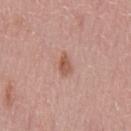Impression:
The lesion was photographed on a routine skin check and not biopsied; there is no pathology result.
Image and clinical context:
The recorded lesion diameter is about 3 mm. A male subject aged 43–47. This image is a 15 mm lesion crop taken from a total-body photograph. The total-body-photography lesion software estimated a lesion area of about 4 mm², an outline eccentricity of about 0.8 (0 = round, 1 = elongated), and two-axis asymmetry of about 0.25. The software also gave an average lesion color of about L≈56 a*≈23 b*≈28 (CIELAB), a lesion–skin lightness drop of about 10, and a lesion-to-skin contrast of about 7 (normalized; higher = more distinct). And it measured a border-irregularity index near 2.5/10, a color-variation rating of about 3/10, and radial color variation of about 1. From the chest.